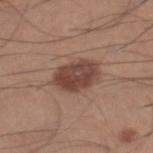notes: total-body-photography surveillance lesion; no biopsy
subject: male, in their mid-30s
diameter: about 5 mm
site: the right lower leg
imaging modality: ~15 mm crop, total-body skin-cancer survey